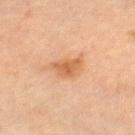Q: Was a biopsy performed?
A: imaged on a skin check; not biopsied
Q: Patient demographics?
A: female, aged approximately 70
Q: What is the imaging modality?
A: total-body-photography crop, ~15 mm field of view
Q: Where on the body is the lesion?
A: the leg